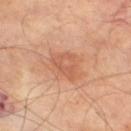{"biopsy_status": "not biopsied; imaged during a skin examination", "patient": {"sex": "male", "age_approx": 70}, "image": {"source": "total-body photography crop", "field_of_view_mm": 15}, "site": "right thigh"}